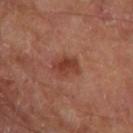notes: no biopsy performed (imaged during a skin exam); lesion diameter: ≈3 mm; acquisition: 15 mm crop, total-body photography; TBP lesion metrics: a border-irregularity rating of about 3/10, internal color variation of about 2.5 on a 0–10 scale, and a peripheral color-asymmetry measure near 1; tile lighting: cross-polarized illumination; subject: male, about 70 years old; body site: the left thigh.